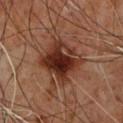Notes:
– biopsy status · imaged on a skin check; not biopsied
– illumination · cross-polarized
– subject · male, roughly 60 years of age
– body site · the upper back
– image · 15 mm crop, total-body photography
– lesion diameter · about 5 mm